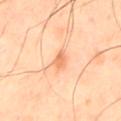{"biopsy_status": "not biopsied; imaged during a skin examination"}